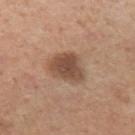Impression: Recorded during total-body skin imaging; not selected for excision or biopsy. Acquisition and patient details: The lesion is located on the left upper arm. The tile uses white-light illumination. The recorded lesion diameter is about 4 mm. The patient is a male roughly 40 years of age. This image is a 15 mm lesion crop taken from a total-body photograph.